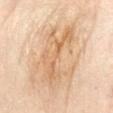workup: catalogued during a skin exam; not biopsied | imaging modality: total-body-photography crop, ~15 mm field of view | image-analysis metrics: an average lesion color of about L≈65 a*≈18 b*≈36 (CIELAB) and a normalized lesion–skin contrast near 6 | subject: female, aged approximately 50 | illumination: cross-polarized | lesion size: ~8 mm (longest diameter) | body site: the left forearm.